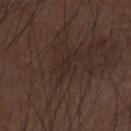notes: catalogued during a skin exam; not biopsied | imaging modality: ~15 mm tile from a whole-body skin photo | subject: male, aged 73 to 77 | lesion diameter: ~4 mm (longest diameter) | illumination: white-light | body site: the right forearm.